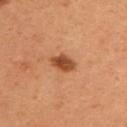{
  "lesion_size": {
    "long_diameter_mm_approx": 3.0
  },
  "site": "upper back",
  "automated_metrics": {
    "area_mm2_approx": 5.0,
    "eccentricity": 0.8,
    "shape_asymmetry": 0.25,
    "border_irregularity_0_10": 2.5,
    "color_variation_0_10": 4.5,
    "nevus_likeness_0_100": 95,
    "lesion_detection_confidence_0_100": 100
  },
  "patient": {
    "sex": "female",
    "age_approx": 40
  },
  "lighting": "cross-polarized",
  "image": {
    "source": "total-body photography crop",
    "field_of_view_mm": 15
  }
}